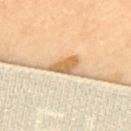The subject is a female aged 58–62. The lesion is on the upper back. A 15 mm close-up tile from a total-body photography series done for melanoma screening.